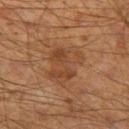Assessment: Part of a total-body skin-imaging series; this lesion was reviewed on a skin check and was not flagged for biopsy. Background: The subject is a male approximately 60 years of age. The lesion is located on the left thigh. The recorded lesion diameter is about 5 mm. This image is a 15 mm lesion crop taken from a total-body photograph. Imaged with cross-polarized lighting.